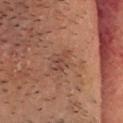{
  "lesion_size": {
    "long_diameter_mm_approx": 2.5
  },
  "lighting": "cross-polarized",
  "patient": {
    "sex": "male",
    "age_approx": 45
  },
  "image": {
    "source": "total-body photography crop",
    "field_of_view_mm": 15
  },
  "site": "head or neck"
}